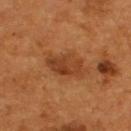Clinical impression:
No biopsy was performed on this lesion — it was imaged during a full skin examination and was not determined to be concerning.
Image and clinical context:
A male patient, aged 53–57. Cropped from a whole-body photographic skin survey; the tile spans about 15 mm. Located on the upper back.